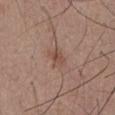Clinical impression:
This lesion was catalogued during total-body skin photography and was not selected for biopsy.
Acquisition and patient details:
The patient is a male aged 53 to 57. A 15 mm crop from a total-body photograph taken for skin-cancer surveillance. The lesion is on the chest. Approximately 2.5 mm at its widest.